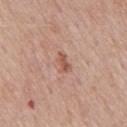<record>
  <biopsy_status>not biopsied; imaged during a skin examination</biopsy_status>
  <patient>
    <sex>male</sex>
    <age_approx>75</age_approx>
  </patient>
  <site>back</site>
  <image>
    <source>total-body photography crop</source>
    <field_of_view_mm>15</field_of_view_mm>
  </image>
</record>